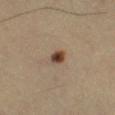Q: Was a biopsy performed?
A: imaged on a skin check; not biopsied
Q: How large is the lesion?
A: ≈2 mm
Q: What kind of image is this?
A: 15 mm crop, total-body photography
Q: What lighting was used for the tile?
A: cross-polarized illumination
Q: Lesion location?
A: the leg
Q: Who is the patient?
A: male, aged approximately 65